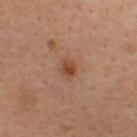Q: Is there a histopathology result?
A: imaged on a skin check; not biopsied
Q: Patient demographics?
A: male, aged 33–37
Q: What kind of image is this?
A: ~15 mm crop, total-body skin-cancer survey
Q: Lesion size?
A: about 2.5 mm
Q: What did automated image analysis measure?
A: a lesion area of about 4 mm² and two-axis asymmetry of about 0.25; a lesion color around L≈44 a*≈21 b*≈31 in CIELAB, roughly 9 lightness units darker than nearby skin, and a normalized border contrast of about 7.5; a classifier nevus-likeness of about 55/100 and lesion-presence confidence of about 100/100
Q: What is the anatomic site?
A: the upper back
Q: What lighting was used for the tile?
A: cross-polarized